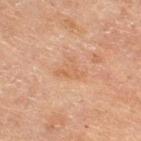Assessment: This lesion was catalogued during total-body skin photography and was not selected for biopsy. Image and clinical context: A roughly 15 mm field-of-view crop from a total-body skin photograph. From the right thigh. Automated tile analysis of the lesion measured a lesion color around L≈53 a*≈19 b*≈32 in CIELAB and a normalized lesion–skin contrast near 4.5. Longest diameter approximately 3.5 mm. The subject is a female approximately 55 years of age.